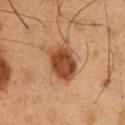Clinical impression:
Recorded during total-body skin imaging; not selected for excision or biopsy.
Context:
The lesion is located on the chest. This is a cross-polarized tile. A lesion tile, about 15 mm wide, cut from a 3D total-body photograph. The subject is a male aged around 50.